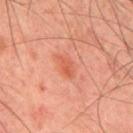The lesion is located on the mid back. A male patient approximately 45 years of age. A 15 mm close-up tile from a total-body photography series done for melanoma screening.